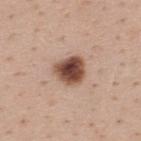<record>
  <biopsy_status>not biopsied; imaged during a skin examination</biopsy_status>
  <lesion_size>
    <long_diameter_mm_approx>3.5</long_diameter_mm_approx>
  </lesion_size>
  <site>upper back</site>
  <lighting>white-light</lighting>
  <automated_metrics>
    <cielab_L>49</cielab_L>
    <cielab_a>20</cielab_a>
    <cielab_b>27</cielab_b>
    <border_irregularity_0_10>2.0</border_irregularity_0_10>
    <color_variation_0_10>7.0</color_variation_0_10>
  </automated_metrics>
  <patient>
    <sex>male</sex>
    <age_approx>40</age_approx>
  </patient>
  <image>
    <source>total-body photography crop</source>
    <field_of_view_mm>15</field_of_view_mm>
  </image>
</record>